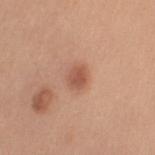Clinical impression:
No biopsy was performed on this lesion — it was imaged during a full skin examination and was not determined to be concerning.